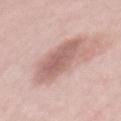Part of a total-body skin-imaging series; this lesion was reviewed on a skin check and was not flagged for biopsy.
A female patient aged 58 to 62.
A roughly 15 mm field-of-view crop from a total-body skin photograph.
From the mid back.